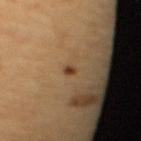The lesion was tiled from a total-body skin photograph and was not biopsied.
A 15 mm close-up extracted from a 3D total-body photography capture.
A female subject, in their mid- to late 60s.
This is a cross-polarized tile.
On the upper back.